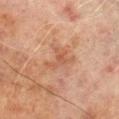{"biopsy_status": "not biopsied; imaged during a skin examination", "lesion_size": {"long_diameter_mm_approx": 4.0}, "patient": {"sex": "male", "age_approx": 70}, "site": "right lower leg", "image": {"source": "total-body photography crop", "field_of_view_mm": 15}}